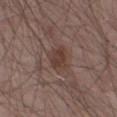follow-up: imaged on a skin check; not biopsied
tile lighting: white-light
site: the left thigh
patient: male, aged around 65
imaging modality: ~15 mm tile from a whole-body skin photo
size: ≈3 mm
image-analysis metrics: a border-irregularity rating of about 2.5/10, a color-variation rating of about 2/10, and radial color variation of about 0.5